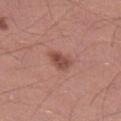This lesion was catalogued during total-body skin photography and was not selected for biopsy. A lesion tile, about 15 mm wide, cut from a 3D total-body photograph. Located on the right lower leg. The tile uses white-light illumination. Automated tile analysis of the lesion measured an area of roughly 5 mm², an outline eccentricity of about 0.75 (0 = round, 1 = elongated), and two-axis asymmetry of about 0.25. The analysis additionally found a lesion color around L≈48 a*≈24 b*≈26 in CIELAB and a lesion–skin lightness drop of about 11. It also reported border irregularity of about 2.5 on a 0–10 scale, a color-variation rating of about 3/10, and peripheral color asymmetry of about 1. A male patient approximately 30 years of age.